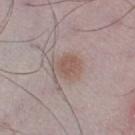Imaged during a routine full-body skin examination; the lesion was not biopsied and no histopathology is available.
Captured under white-light illumination.
The subject is a male roughly 50 years of age.
This image is a 15 mm lesion crop taken from a total-body photograph.
The lesion is located on the left thigh.
Automated tile analysis of the lesion measured a lesion area of about 7.5 mm² and a shape-asymmetry score of about 0.25 (0 = symmetric). It also reported internal color variation of about 2.5 on a 0–10 scale and a peripheral color-asymmetry measure near 1. The software also gave an automated nevus-likeness rating near 95 out of 100.